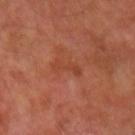No biopsy was performed on this lesion — it was imaged during a full skin examination and was not determined to be concerning. The tile uses cross-polarized illumination. This image is a 15 mm lesion crop taken from a total-body photograph. From the left forearm. The subject is a male in their 50s.